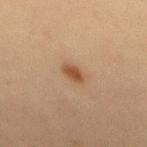• notes · no biopsy performed (imaged during a skin exam)
• lesion size · ≈2.5 mm
• acquisition · ~15 mm tile from a whole-body skin photo
• anatomic site · the back
• tile lighting · cross-polarized illumination
• patient · male, aged 58–62